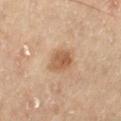Q: Was a biopsy performed?
A: no biopsy performed (imaged during a skin exam)
Q: What lighting was used for the tile?
A: cross-polarized
Q: What kind of image is this?
A: ~15 mm crop, total-body skin-cancer survey
Q: Lesion location?
A: the leg
Q: Patient demographics?
A: male, approximately 70 years of age